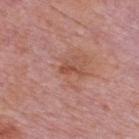This lesion was catalogued during total-body skin photography and was not selected for biopsy.
Cropped from a total-body skin-imaging series; the visible field is about 15 mm.
On the back.
The total-body-photography lesion software estimated a footprint of about 2.5 mm² and two-axis asymmetry of about 0.45. The software also gave roughly 9 lightness units darker than nearby skin and a lesion-to-skin contrast of about 7 (normalized; higher = more distinct). It also reported an automated nevus-likeness rating near 0 out of 100 and a lesion-detection confidence of about 100/100.
The subject is a male approximately 75 years of age.